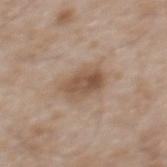biopsy status: no biopsy performed (imaged during a skin exam); subject: male, aged around 50; imaging modality: ~15 mm tile from a whole-body skin photo; anatomic site: the mid back.